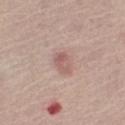  biopsy_status: not biopsied; imaged during a skin examination
  site: leg
  lighting: white-light
  image:
    source: total-body photography crop
    field_of_view_mm: 15
  lesion_size:
    long_diameter_mm_approx: 2.5
  patient:
    sex: male
    age_approx: 65
  automated_metrics:
    area_mm2_approx: 3.0
    eccentricity: 0.8
    shape_asymmetry: 0.5
    nevus_likeness_0_100: 55
    lesion_detection_confidence_0_100: 100